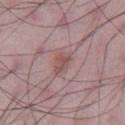Imaged during a routine full-body skin examination; the lesion was not biopsied and no histopathology is available.
A lesion tile, about 15 mm wide, cut from a 3D total-body photograph.
On the leg.
This is a white-light tile.
The recorded lesion diameter is about 3.5 mm.
A male patient, in their 50s.